A close-up tile cropped from a whole-body skin photograph, about 15 mm across; Automated tile analysis of the lesion measured an outline eccentricity of about 0.85 (0 = round, 1 = elongated) and a symmetry-axis asymmetry near 0.35. The software also gave a border-irregularity index near 3/10, a within-lesion color-variation index near 0/10, and peripheral color asymmetry of about 0. And it measured an automated nevus-likeness rating near 0 out of 100 and lesion-presence confidence of about 100/100; from the upper back; about 1.5 mm across; a male patient about 50 years old.
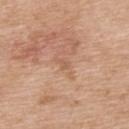Q: What is the histopathologic diagnosis?
A: an invasive melanoma, Breslow thickness 0.3 mm